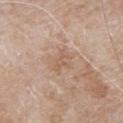Recorded during total-body skin imaging; not selected for excision or biopsy.
The lesion's longest dimension is about 2.5 mm.
A male subject aged 78–82.
A region of skin cropped from a whole-body photographic capture, roughly 15 mm wide.
From the chest.